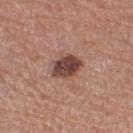No biopsy was performed on this lesion — it was imaged during a full skin examination and was not determined to be concerning. On the right thigh. The patient is a female roughly 60 years of age. A region of skin cropped from a whole-body photographic capture, roughly 15 mm wide.